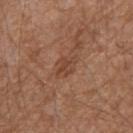  biopsy_status: not biopsied; imaged during a skin examination
  image:
    source: total-body photography crop
    field_of_view_mm: 15
  site: right upper arm
  patient:
    sex: male
    age_approx: 55
  lighting: white-light
  lesion_size:
    long_diameter_mm_approx: 2.5